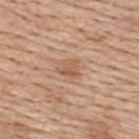Clinical impression: This lesion was catalogued during total-body skin photography and was not selected for biopsy. Image and clinical context: An algorithmic analysis of the crop reported an eccentricity of roughly 0.5 and a shape-asymmetry score of about 0.4 (0 = symmetric). The software also gave border irregularity of about 3.5 on a 0–10 scale, a within-lesion color-variation index near 3.5/10, and radial color variation of about 1. It also reported a nevus-likeness score of about 0/100 and a lesion-detection confidence of about 100/100. This image is a 15 mm lesion crop taken from a total-body photograph. From the upper back. A male patient aged approximately 60.